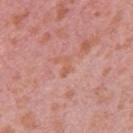A female patient, aged 38–42. The total-body-photography lesion software estimated a mean CIELAB color near L≈59 a*≈26 b*≈31, roughly 6 lightness units darker than nearby skin, and a normalized lesion–skin contrast near 5.5. On the right upper arm. The tile uses white-light illumination. A 15 mm crop from a total-body photograph taken for skin-cancer surveillance. Measured at roughly 2.5 mm in maximum diameter.